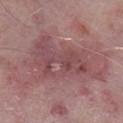Q: Is there a histopathology result?
A: no biopsy performed (imaged during a skin exam)
Q: How was the tile lit?
A: white-light
Q: What kind of image is this?
A: total-body-photography crop, ~15 mm field of view
Q: Lesion size?
A: ≈9 mm
Q: Automated lesion metrics?
A: an area of roughly 30 mm² and a shape eccentricity near 0.85; a border-irregularity index near 6/10, a within-lesion color-variation index near 6/10, and a peripheral color-asymmetry measure near 2; a classifier nevus-likeness of about 0/100 and a detector confidence of about 50 out of 100 that the crop contains a lesion
Q: Lesion location?
A: the right lower leg
Q: What are the patient's age and sex?
A: male, aged approximately 75